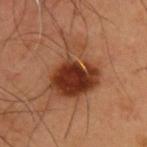Assessment: No biopsy was performed on this lesion — it was imaged during a full skin examination and was not determined to be concerning. Image and clinical context: The lesion-visualizer software estimated an area of roughly 24 mm², an eccentricity of roughly 0.55, and a symmetry-axis asymmetry near 0.4. It also reported an average lesion color of about L≈37 a*≈24 b*≈31 (CIELAB) and a normalized border contrast of about 11. Captured under cross-polarized illumination. A male patient, aged approximately 55. Approximately 7 mm at its widest. On the upper back. A lesion tile, about 15 mm wide, cut from a 3D total-body photograph.